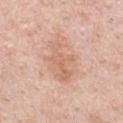No biopsy was performed on this lesion — it was imaged during a full skin examination and was not determined to be concerning. An algorithmic analysis of the crop reported a border-irregularity rating of about 5.5/10 and radial color variation of about 1. A male patient, in their 50s. From the chest. Imaged with white-light lighting. Cropped from a whole-body photographic skin survey; the tile spans about 15 mm.